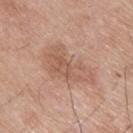Notes:
* follow-up · no biopsy performed (imaged during a skin exam)
* site · the back
* lesion size · ~6.5 mm (longest diameter)
* imaging modality · ~15 mm crop, total-body skin-cancer survey
* subject · male, aged approximately 75